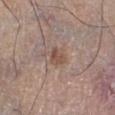<case>
  <biopsy_status>not biopsied; imaged during a skin examination</biopsy_status>
  <patient>
    <sex>male</sex>
    <age_approx>70</age_approx>
  </patient>
  <lesion_size>
    <long_diameter_mm_approx>3.0</long_diameter_mm_approx>
  </lesion_size>
  <site>right lower leg</site>
  <lighting>white-light</lighting>
  <automated_metrics>
    <eccentricity>0.7</eccentricity>
    <cielab_L>50</cielab_L>
    <cielab_a>17</cielab_a>
    <cielab_b>25</cielab_b>
    <vs_skin_contrast_norm>6.5</vs_skin_contrast_norm>
    <border_irregularity_0_10>2.5</border_irregularity_0_10>
    <color_variation_0_10>3.0</color_variation_0_10>
    <peripheral_color_asymmetry>1.0</peripheral_color_asymmetry>
  </automated_metrics>
  <image>
    <source>total-body photography crop</source>
    <field_of_view_mm>15</field_of_view_mm>
  </image>
</case>